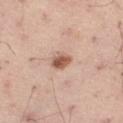This lesion was catalogued during total-body skin photography and was not selected for biopsy.
This image is a 15 mm lesion crop taken from a total-body photograph.
This is a white-light tile.
The lesion-visualizer software estimated a lesion area of about 4.5 mm², an eccentricity of roughly 0.6, and a shape-asymmetry score of about 0.25 (0 = symmetric). The software also gave a mean CIELAB color near L≈58 a*≈21 b*≈28, a lesion–skin lightness drop of about 14, and a normalized border contrast of about 9.
The subject is a male about 55 years old.
Located on the leg.